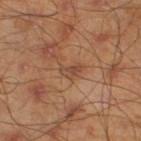Cropped from a total-body skin-imaging series; the visible field is about 15 mm. Imaged with cross-polarized lighting. An algorithmic analysis of the crop reported an automated nevus-likeness rating near 0 out of 100 and a detector confidence of about 100 out of 100 that the crop contains a lesion. The patient is a male about 45 years old. The lesion is located on the left lower leg.